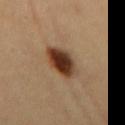- follow-up · catalogued during a skin exam; not biopsied
- lesion size · about 4.5 mm
- imaging modality · ~15 mm crop, total-body skin-cancer survey
- TBP lesion metrics · a lesion area of about 11 mm² and a shape eccentricity near 0.75
- patient · male, aged approximately 50
- site · the mid back